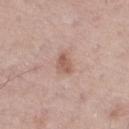Impression: Part of a total-body skin-imaging series; this lesion was reviewed on a skin check and was not flagged for biopsy. Background: Measured at roughly 2.5 mm in maximum diameter. The lesion is located on the right thigh. An algorithmic analysis of the crop reported an area of roughly 3.5 mm² and a symmetry-axis asymmetry near 0.3. The software also gave a mean CIELAB color near L≈57 a*≈20 b*≈27 and a normalized border contrast of about 7. It also reported a border-irregularity rating of about 3/10 and peripheral color asymmetry of about 0.5. It also reported a nevus-likeness score of about 50/100 and lesion-presence confidence of about 100/100. The patient is a male aged 53–57. Captured under white-light illumination. A 15 mm close-up extracted from a 3D total-body photography capture.